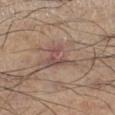automated_metrics:
  cielab_L: 49
  cielab_a: 18
  cielab_b: 23
  vs_skin_contrast_norm: 7.0
lesion_size:
  long_diameter_mm_approx: 4.0
image:
  source: total-body photography crop
  field_of_view_mm: 15
lighting: white-light
patient:
  sex: male
  age_approx: 55
site: left lower leg